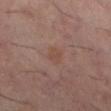Imaged during a routine full-body skin examination; the lesion was not biopsied and no histopathology is available.
The patient is a male in their 60s.
The total-body-photography lesion software estimated a lesion area of about 4.5 mm² and a shape eccentricity near 0.55. The software also gave a nevus-likeness score of about 0/100.
Located on the leg.
A close-up tile cropped from a whole-body skin photograph, about 15 mm across.
This is a cross-polarized tile.
Measured at roughly 2.5 mm in maximum diameter.